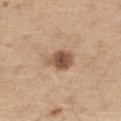Q: Is there a histopathology result?
A: total-body-photography surveillance lesion; no biopsy
Q: What is the lesion's diameter?
A: about 3.5 mm
Q: What is the imaging modality?
A: 15 mm crop, total-body photography
Q: Who is the patient?
A: male, aged approximately 70
Q: Lesion location?
A: the right thigh
Q: How was the tile lit?
A: white-light